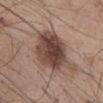Background: The lesion-visualizer software estimated a lesion color around L≈43 a*≈17 b*≈23 in CIELAB and a lesion–skin lightness drop of about 15. It also reported border irregularity of about 3 on a 0–10 scale, a within-lesion color-variation index near 6.5/10, and peripheral color asymmetry of about 2.5. It also reported an automated nevus-likeness rating near 90 out of 100. A male patient roughly 55 years of age. The tile uses white-light illumination. The lesion is located on the chest. A close-up tile cropped from a whole-body skin photograph, about 15 mm across.